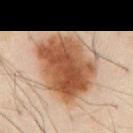<record>
<biopsy_status>not biopsied; imaged during a skin examination</biopsy_status>
<lesion_size>
  <long_diameter_mm_approx>8.5</long_diameter_mm_approx>
</lesion_size>
<image>
  <source>total-body photography crop</source>
  <field_of_view_mm>15</field_of_view_mm>
</image>
<patient>
  <sex>male</sex>
  <age_approx>55</age_approx>
</patient>
<site>abdomen</site>
<lighting>cross-polarized</lighting>
</record>